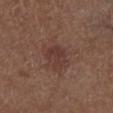Impression: Imaged during a routine full-body skin examination; the lesion was not biopsied and no histopathology is available. Context: Approximately 3 mm at its widest. Located on the right lower leg. The tile uses white-light illumination. Cropped from a total-body skin-imaging series; the visible field is about 15 mm. A male patient aged 73–77.